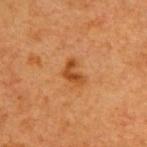A female subject, roughly 40 years of age. On the upper back. Longest diameter approximately 3 mm. This is a cross-polarized tile. This image is a 15 mm lesion crop taken from a total-body photograph.